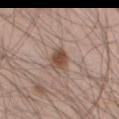Notes:
– notes · catalogued during a skin exam; not biopsied
– location · the left thigh
– subject · male, aged 43 to 47
– lighting · white-light illumination
– imaging modality · total-body-photography crop, ~15 mm field of view
– size · ~3 mm (longest diameter)